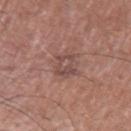Imaged during a routine full-body skin examination; the lesion was not biopsied and no histopathology is available.
The lesion's longest dimension is about 3.5 mm.
Located on the left upper arm.
Automated image analysis of the tile measured a lesion color around L≈47 a*≈20 b*≈22 in CIELAB, a lesion–skin lightness drop of about 8, and a normalized border contrast of about 6. The software also gave border irregularity of about 4 on a 0–10 scale, a within-lesion color-variation index near 5.5/10, and a peripheral color-asymmetry measure near 2.
This is a white-light tile.
A lesion tile, about 15 mm wide, cut from a 3D total-body photograph.
A male patient in their mid-60s.